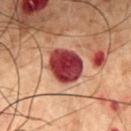Captured during whole-body skin photography for melanoma surveillance; the lesion was not biopsied. On the mid back. A male patient roughly 50 years of age. A roughly 15 mm field-of-view crop from a total-body skin photograph.